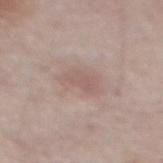Clinical impression:
This lesion was catalogued during total-body skin photography and was not selected for biopsy.
Clinical summary:
The lesion is located on the abdomen. Measured at roughly 4 mm in maximum diameter. This is a white-light tile. A male patient roughly 55 years of age. Cropped from a whole-body photographic skin survey; the tile spans about 15 mm.